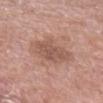notes: catalogued during a skin exam; not biopsied
illumination: white-light illumination
diameter: ≈4.5 mm
image: ~15 mm tile from a whole-body skin photo
site: the arm
subject: male, aged around 70